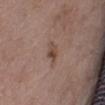<tbp_lesion>
  <lighting>white-light</lighting>
  <automated_metrics>
    <eccentricity>0.75</eccentricity>
    <shape_asymmetry>0.25</shape_asymmetry>
    <vs_skin_darker_L>8.0</vs_skin_darker_L>
    <vs_skin_contrast_norm>6.5</vs_skin_contrast_norm>
  </automated_metrics>
  <site>abdomen</site>
  <lesion_size>
    <long_diameter_mm_approx>2.5</long_diameter_mm_approx>
  </lesion_size>
  <image>
    <source>total-body photography crop</source>
    <field_of_view_mm>15</field_of_view_mm>
  </image>
  <patient>
    <sex>female</sex>
    <age_approx>70</age_approx>
  </patient>
</tbp_lesion>